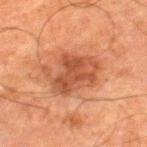Imaged during a routine full-body skin examination; the lesion was not biopsied and no histopathology is available. Cropped from a total-body skin-imaging series; the visible field is about 15 mm. This is a cross-polarized tile. On the leg. A male subject aged around 80. Approximately 5.5 mm at its widest. Automated image analysis of the tile measured a lesion area of about 17 mm² and a shape-asymmetry score of about 0.35 (0 = symmetric). It also reported a mean CIELAB color near L≈41 a*≈23 b*≈30, about 9 CIELAB-L* units darker than the surrounding skin, and a normalized border contrast of about 7.5. The software also gave a border-irregularity rating of about 5/10, a within-lesion color-variation index near 3.5/10, and a peripheral color-asymmetry measure near 1.5.